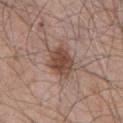Clinical impression: This lesion was catalogued during total-body skin photography and was not selected for biopsy. Image and clinical context: On the chest. A 15 mm close-up extracted from a 3D total-body photography capture. Captured under white-light illumination. A male subject aged 78 to 82. About 4 mm across.